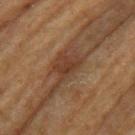No biopsy was performed on this lesion — it was imaged during a full skin examination and was not determined to be concerning.
The recorded lesion diameter is about 3 mm.
Automated tile analysis of the lesion measured border irregularity of about 4.5 on a 0–10 scale, a within-lesion color-variation index near 1/10, and radial color variation of about 0.5. And it measured a nevus-likeness score of about 25/100 and lesion-presence confidence of about 95/100.
Located on the back.
A close-up tile cropped from a whole-body skin photograph, about 15 mm across.
This is a cross-polarized tile.
The patient is a male approximately 65 years of age.